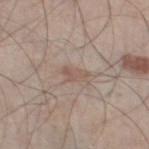Assessment: Imaged during a routine full-body skin examination; the lesion was not biopsied and no histopathology is available. Context: A male subject roughly 60 years of age. Measured at roughly 3 mm in maximum diameter. The tile uses white-light illumination. An algorithmic analysis of the crop reported an area of roughly 4 mm² and two-axis asymmetry of about 0.35. The lesion is located on the left thigh. A 15 mm crop from a total-body photograph taken for skin-cancer surveillance.